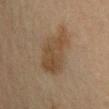  biopsy_status: not biopsied; imaged during a skin examination
  patient:
    sex: female
    age_approx: 55
  site: leg
  image:
    source: total-body photography crop
    field_of_view_mm: 15